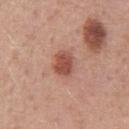<lesion>
<biopsy_status>not biopsied; imaged during a skin examination</biopsy_status>
<site>chest</site>
<patient>
  <sex>male</sex>
  <age_approx>50</age_approx>
</patient>
<lesion_size>
  <long_diameter_mm_approx>3.0</long_diameter_mm_approx>
</lesion_size>
<automated_metrics>
  <cielab_L>51</cielab_L>
  <cielab_a>26</cielab_a>
  <cielab_b>29</cielab_b>
  <vs_skin_darker_L>12.0</vs_skin_darker_L>
  <vs_skin_contrast_norm>8.5</vs_skin_contrast_norm>
  <border_irregularity_0_10>1.5</border_irregularity_0_10>
  <peripheral_color_asymmetry>1.0</peripheral_color_asymmetry>
  <nevus_likeness_0_100>90</nevus_likeness_0_100>
  <lesion_detection_confidence_0_100>100</lesion_detection_confidence_0_100>
</automated_metrics>
<image>
  <source>total-body photography crop</source>
  <field_of_view_mm>15</field_of_view_mm>
</image>
</lesion>